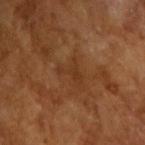Clinical impression: Imaged during a routine full-body skin examination; the lesion was not biopsied and no histopathology is available. Background: The patient is a male roughly 65 years of age. Captured under cross-polarized illumination. A lesion tile, about 15 mm wide, cut from a 3D total-body photograph.